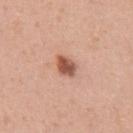Clinical summary:
The patient is a male aged 53–57. A close-up tile cropped from a whole-body skin photograph, about 15 mm across. Captured under white-light illumination. The recorded lesion diameter is about 3 mm. Located on the abdomen. Automated image analysis of the tile measured roughly 15 lightness units darker than nearby skin and a lesion-to-skin contrast of about 10 (normalized; higher = more distinct). The analysis additionally found peripheral color asymmetry of about 1.